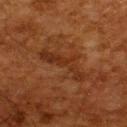{"lighting": "cross-polarized", "lesion_size": {"long_diameter_mm_approx": 6.0}, "image": {"source": "total-body photography crop", "field_of_view_mm": 15}, "site": "back", "patient": {"sex": "male", "age_approx": 65}}